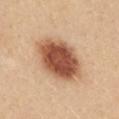Case summary:
– workup — imaged on a skin check; not biopsied
– image source — 15 mm crop, total-body photography
– subject — female, aged 23 to 27
– location — the upper back